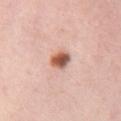Part of a total-body skin-imaging series; this lesion was reviewed on a skin check and was not flagged for biopsy. Cropped from a whole-body photographic skin survey; the tile spans about 15 mm. The lesion is on the chest. A female patient about 35 years old.